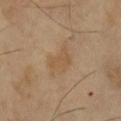biopsy status: catalogued during a skin exam; not biopsied | illumination: cross-polarized illumination | size: ~3.5 mm (longest diameter) | imaging modality: ~15 mm tile from a whole-body skin photo | subject: female, about 70 years old | location: the right lower leg | automated lesion analysis: a footprint of about 7 mm² and an eccentricity of roughly 0.65; a border-irregularity index near 3/10 and radial color variation of about 0.5; a nevus-likeness score of about 10/100 and a lesion-detection confidence of about 100/100.